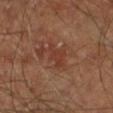This lesion was catalogued during total-body skin photography and was not selected for biopsy.
Measured at roughly 3.5 mm in maximum diameter.
A lesion tile, about 15 mm wide, cut from a 3D total-body photograph.
The total-body-photography lesion software estimated an area of roughly 4 mm², an eccentricity of roughly 0.9, and two-axis asymmetry of about 0.35. It also reported a lesion color around L≈35 a*≈23 b*≈28 in CIELAB, roughly 6 lightness units darker than nearby skin, and a normalized lesion–skin contrast near 5.5. And it measured peripheral color asymmetry of about 0.5.
The lesion is located on the leg.
A male patient, aged 58–62.
Captured under cross-polarized illumination.